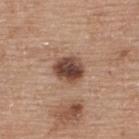Clinical impression: The lesion was tiled from a total-body skin photograph and was not biopsied. Image and clinical context: The lesion is located on the upper back. This image is a 15 mm lesion crop taken from a total-body photograph. A female patient aged 58–62.